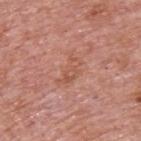Notes:
– follow-up: total-body-photography surveillance lesion; no biopsy
– illumination: white-light illumination
– image: 15 mm crop, total-body photography
– subject: male, in their mid-70s
– lesion diameter: about 4 mm
– TBP lesion metrics: an average lesion color of about L≈55 a*≈25 b*≈31 (CIELAB), roughly 6 lightness units darker than nearby skin, and a normalized lesion–skin contrast near 5; a lesion-detection confidence of about 100/100
– site: the upper back